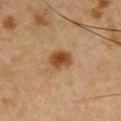A 15 mm close-up extracted from a 3D total-body photography capture.
This is a cross-polarized tile.
The total-body-photography lesion software estimated a lesion area of about 7.5 mm², an outline eccentricity of about 0.55 (0 = round, 1 = elongated), and a shape-asymmetry score of about 0.2 (0 = symmetric). The software also gave a border-irregularity index near 1.5/10 and a within-lesion color-variation index near 5.5/10.
A male patient, aged around 55.
The lesion is on the front of the torso.
Approximately 3 mm at its widest.